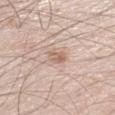biopsy status: no biopsy performed (imaged during a skin exam)
subject: male, approximately 60 years of age
imaging modality: 15 mm crop, total-body photography
lighting: white-light illumination
lesion size: ~2.5 mm (longest diameter)
anatomic site: the left lower leg